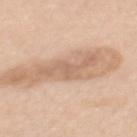{"site": "mid back", "image": {"source": "total-body photography crop", "field_of_view_mm": 15}, "patient": {"sex": "female", "age_approx": 60}, "automated_metrics": {"eccentricity": 0.95, "shape_asymmetry": 0.55, "border_irregularity_0_10": 9.0, "lesion_detection_confidence_0_100": 60}}